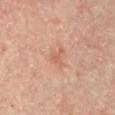<record>
  <patient>
    <sex>male</sex>
    <age_approx>65</age_approx>
  </patient>
  <site>abdomen</site>
  <image>
    <source>total-body photography crop</source>
    <field_of_view_mm>15</field_of_view_mm>
  </image>
  <automated_metrics>
    <cielab_L>59</cielab_L>
    <cielab_a>23</cielab_a>
    <cielab_b>32</cielab_b>
    <vs_skin_darker_L>7.0</vs_skin_darker_L>
    <vs_skin_contrast_norm>5.0</vs_skin_contrast_norm>
    <border_irregularity_0_10>6.0</border_irregularity_0_10>
    <color_variation_0_10>1.0</color_variation_0_10>
  </automated_metrics>
  <lesion_size>
    <long_diameter_mm_approx>2.5</long_diameter_mm_approx>
  </lesion_size>
</record>